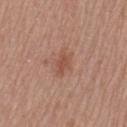Captured during whole-body skin photography for melanoma surveillance; the lesion was not biopsied.